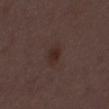The lesion was tiled from a total-body skin photograph and was not biopsied.
The recorded lesion diameter is about 3.5 mm.
Automated image analysis of the tile measured a footprint of about 5.5 mm². And it measured a lesion color around L≈27 a*≈15 b*≈19 in CIELAB, about 5 CIELAB-L* units darker than the surrounding skin, and a normalized lesion–skin contrast near 7. The analysis additionally found a within-lesion color-variation index near 2.5/10 and a peripheral color-asymmetry measure near 0.5. The software also gave lesion-presence confidence of about 100/100.
A male subject, about 50 years old.
A 15 mm close-up tile from a total-body photography series done for melanoma screening.